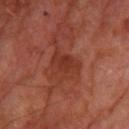follow-up: no biopsy performed (imaged during a skin exam)
subject: male, in their 70s
imaging modality: 15 mm crop, total-body photography
body site: the right thigh
lighting: cross-polarized illumination
TBP lesion metrics: a footprint of about 9 mm², an eccentricity of roughly 0.6, and two-axis asymmetry of about 0.3; an average lesion color of about L≈35 a*≈27 b*≈30 (CIELAB) and a lesion-to-skin contrast of about 6.5 (normalized; higher = more distinct); lesion-presence confidence of about 100/100
lesion size: ~4 mm (longest diameter)